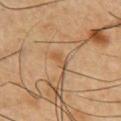  patient:
    sex: male
    age_approx: 55
  image:
    source: total-body photography crop
    field_of_view_mm: 15
  automated_metrics:
    cielab_L: 52
    cielab_a: 19
    cielab_b: 37
    vs_skin_darker_L: 7.0
    vs_skin_contrast_norm: 6.0
    color_variation_0_10: 0.0
    peripheral_color_asymmetry: 0.0
    nevus_likeness_0_100: 10
    lesion_detection_confidence_0_100: 75
  site: chest
  lighting: cross-polarized
  lesion_size:
    long_diameter_mm_approx: 2.5